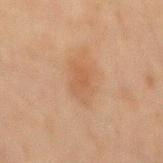notes = no biopsy performed (imaged during a skin exam) | patient = male, roughly 45 years of age | location = the mid back | acquisition = 15 mm crop, total-body photography | lighting = cross-polarized | automated lesion analysis = about 6 CIELAB-L* units darker than the surrounding skin; a border-irregularity index near 3.5/10, internal color variation of about 2 on a 0–10 scale, and peripheral color asymmetry of about 0.5; a nevus-likeness score of about 15/100 and a lesion-detection confidence of about 100/100 | size = about 4 mm.